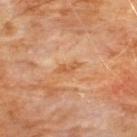No biopsy was performed on this lesion — it was imaged during a full skin examination and was not determined to be concerning. This image is a 15 mm lesion crop taken from a total-body photograph. The patient is a male in their 60s. Captured under cross-polarized illumination. From the chest.